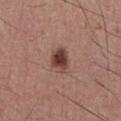Notes:
– workup — total-body-photography surveillance lesion; no biopsy
– tile lighting — white-light
– acquisition — total-body-photography crop, ~15 mm field of view
– size — ~3 mm (longest diameter)
– body site — the chest
– subject — male, aged approximately 40